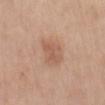Recorded during total-body skin imaging; not selected for excision or biopsy.
The lesion's longest dimension is about 4 mm.
A female patient aged approximately 60.
A lesion tile, about 15 mm wide, cut from a 3D total-body photograph.
From the back.
Captured under white-light illumination.